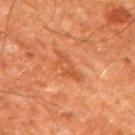The lesion was tiled from a total-body skin photograph and was not biopsied.
On the right upper arm.
A 15 mm crop from a total-body photograph taken for skin-cancer surveillance.
A male patient aged around 60.